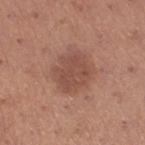follow-up = no biopsy performed (imaged during a skin exam) | location = the right thigh | subject = female, aged around 40 | lesion diameter = ~4 mm (longest diameter) | image = ~15 mm tile from a whole-body skin photo.